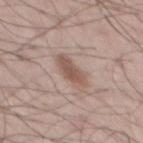| feature | finding |
|---|---|
| workup | catalogued during a skin exam; not biopsied |
| diameter | about 4.5 mm |
| anatomic site | the mid back |
| acquisition | 15 mm crop, total-body photography |
| subject | male, in their mid- to late 40s |
| tile lighting | white-light |